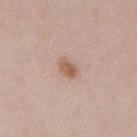follow-up: total-body-photography surveillance lesion; no biopsy
diameter: ~2.5 mm (longest diameter)
patient: female, in their mid- to late 60s
anatomic site: the mid back
image source: ~15 mm crop, total-body skin-cancer survey
tile lighting: white-light
automated lesion analysis: a footprint of about 3.5 mm²; a lesion–skin lightness drop of about 10 and a lesion-to-skin contrast of about 7.5 (normalized; higher = more distinct); a border-irregularity rating of about 2/10, internal color variation of about 2.5 on a 0–10 scale, and peripheral color asymmetry of about 1